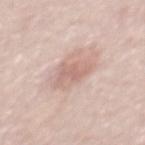Recorded during total-body skin imaging; not selected for excision or biopsy. A 15 mm close-up extracted from a 3D total-body photography capture. Approximately 4 mm at its widest. A male subject about 50 years old. The lesion is located on the mid back.